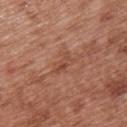Impression:
The lesion was tiled from a total-body skin photograph and was not biopsied.
Acquisition and patient details:
A female subject aged 38–42. From the upper back. Cropped from a whole-body photographic skin survey; the tile spans about 15 mm.